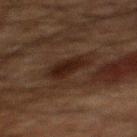Assessment:
Recorded during total-body skin imaging; not selected for excision or biopsy.
Context:
A lesion tile, about 15 mm wide, cut from a 3D total-body photograph. The lesion is on the mid back. Captured under cross-polarized illumination. A male subject aged around 60.